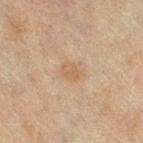Q: Is there a histopathology result?
A: imaged on a skin check; not biopsied
Q: How large is the lesion?
A: about 2.5 mm
Q: Illumination type?
A: cross-polarized illumination
Q: Patient demographics?
A: female, aged approximately 55
Q: What is the anatomic site?
A: the leg
Q: What kind of image is this?
A: total-body-photography crop, ~15 mm field of view